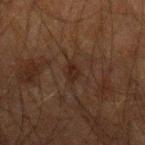Impression:
This lesion was catalogued during total-body skin photography and was not selected for biopsy.